A male subject, approximately 55 years of age · a region of skin cropped from a whole-body photographic capture, roughly 15 mm wide · on the upper back.
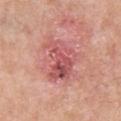Histopathologically confirmed as a superficial basal cell carcinoma.